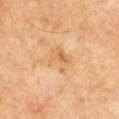image source — total-body-photography crop, ~15 mm field of view | location — the upper back | lesion size — about 3.5 mm | lighting — cross-polarized illumination | subject — male, in their 60s.